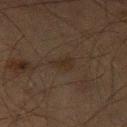Clinical impression:
The lesion was photographed on a routine skin check and not biopsied; there is no pathology result.